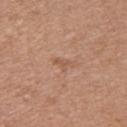Impression:
No biopsy was performed on this lesion — it was imaged during a full skin examination and was not determined to be concerning.
Context:
The lesion is located on the upper back. An algorithmic analysis of the crop reported a footprint of about 2.5 mm² and two-axis asymmetry of about 0.55. The analysis additionally found about 6 CIELAB-L* units darker than the surrounding skin and a lesion-to-skin contrast of about 4.5 (normalized; higher = more distinct). And it measured a border-irregularity index near 5.5/10, internal color variation of about 0 on a 0–10 scale, and peripheral color asymmetry of about 0. Longest diameter approximately 2.5 mm. A female patient in their mid- to late 30s. A 15 mm crop from a total-body photograph taken for skin-cancer surveillance.